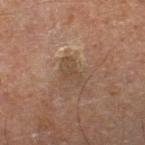Part of a total-body skin-imaging series; this lesion was reviewed on a skin check and was not flagged for biopsy. Cropped from a total-body skin-imaging series; the visible field is about 15 mm. Imaged with cross-polarized lighting. The subject is a male in their mid-60s. About 4.5 mm across. The lesion is on the right lower leg.